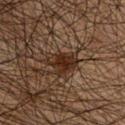Q: What lighting was used for the tile?
A: cross-polarized illumination
Q: How was this image acquired?
A: total-body-photography crop, ~15 mm field of view
Q: How large is the lesion?
A: about 4 mm
Q: Patient demographics?
A: male, in their 60s
Q: What is the anatomic site?
A: the front of the torso